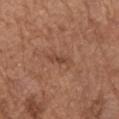Cropped from a total-body skin-imaging series; the visible field is about 15 mm. The lesion is located on the right upper arm. A male subject, aged around 75.